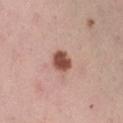Part of a total-body skin-imaging series; this lesion was reviewed on a skin check and was not flagged for biopsy. The lesion is on the right thigh. The subject is a female roughly 35 years of age. Imaged with white-light lighting. The lesion's longest dimension is about 2.5 mm. The lesion-visualizer software estimated a lesion-to-skin contrast of about 11.5 (normalized; higher = more distinct). A close-up tile cropped from a whole-body skin photograph, about 15 mm across.